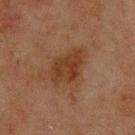The patient is a male approximately 45 years of age.
An algorithmic analysis of the crop reported a shape eccentricity near 0.75 and a shape-asymmetry score of about 0.25 (0 = symmetric). The analysis additionally found a lesion color around L≈30 a*≈18 b*≈27 in CIELAB, about 7 CIELAB-L* units darker than the surrounding skin, and a normalized border contrast of about 7.5. It also reported border irregularity of about 3 on a 0–10 scale, a within-lesion color-variation index near 3/10, and radial color variation of about 1. And it measured a nevus-likeness score of about 5/100 and lesion-presence confidence of about 100/100.
Imaged with cross-polarized lighting.
Located on the upper back.
Cropped from a whole-body photographic skin survey; the tile spans about 15 mm.
Measured at roughly 5 mm in maximum diameter.